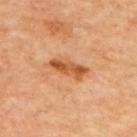Captured during whole-body skin photography for melanoma surveillance; the lesion was not biopsied.
A lesion tile, about 15 mm wide, cut from a 3D total-body photograph.
The patient is approximately 65 years of age.
The lesion is located on the upper back.